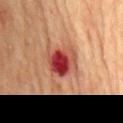Clinical impression:
Recorded during total-body skin imaging; not selected for excision or biopsy.
Background:
A 15 mm crop from a total-body photograph taken for skin-cancer surveillance. A male patient, about 70 years old. The lesion is on the back. This is a cross-polarized tile.